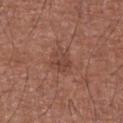Impression: No biopsy was performed on this lesion — it was imaged during a full skin examination and was not determined to be concerning. Context: A 15 mm crop from a total-body photograph taken for skin-cancer surveillance. The subject is a male in their mid-60s. The lesion's longest dimension is about 3 mm. The lesion is located on the chest.